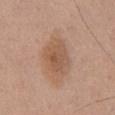Findings:
• follow-up: no biopsy performed (imaged during a skin exam)
• subject: male, approximately 55 years of age
• acquisition: total-body-photography crop, ~15 mm field of view
• image-analysis metrics: a border-irregularity index near 2/10 and internal color variation of about 3.5 on a 0–10 scale; a nevus-likeness score of about 45/100 and a lesion-detection confidence of about 100/100
• site: the chest
• size: ≈5 mm